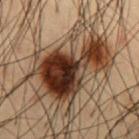* notes · imaged on a skin check; not biopsied
* acquisition · ~15 mm tile from a whole-body skin photo
* patient · male, in their mid- to late 50s
* site · the abdomen
* lesion diameter · ≈8 mm
* tile lighting · cross-polarized